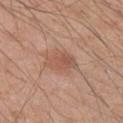Findings:
– lighting — white-light
– body site — the right upper arm
– patient — male, roughly 45 years of age
– acquisition — ~15 mm crop, total-body skin-cancer survey
– diameter — ~4 mm (longest diameter)